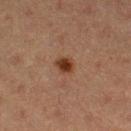| feature | finding |
|---|---|
| acquisition | ~15 mm crop, total-body skin-cancer survey |
| site | the right thigh |
| size | ~2.5 mm (longest diameter) |
| subject | female, aged around 40 |